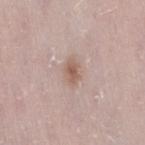<record>
  <biopsy_status>not biopsied; imaged during a skin examination</biopsy_status>
  <lighting>white-light</lighting>
  <patient>
    <sex>female</sex>
    <age_approx>30</age_approx>
  </patient>
  <lesion_size>
    <long_diameter_mm_approx>3.0</long_diameter_mm_approx>
  </lesion_size>
  <image>
    <source>total-body photography crop</source>
    <field_of_view_mm>15</field_of_view_mm>
  </image>
  <site>right thigh</site>
  <automated_metrics>
    <vs_skin_darker_L>10.0</vs_skin_darker_L>
    <border_irregularity_0_10>2.0</border_irregularity_0_10>
    <color_variation_0_10>2.5</color_variation_0_10>
    <peripheral_color_asymmetry>0.5</peripheral_color_asymmetry>
    <nevus_likeness_0_100>60</nevus_likeness_0_100>
    <lesion_detection_confidence_0_100>100</lesion_detection_confidence_0_100>
  </automated_metrics>
</record>